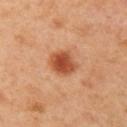follow-up: total-body-photography surveillance lesion; no biopsy
site: the left upper arm
automated metrics: a footprint of about 8 mm², a shape eccentricity near 0.5, and two-axis asymmetry of about 0.2; about 14 CIELAB-L* units darker than the surrounding skin; a border-irregularity rating of about 2/10 and internal color variation of about 4.5 on a 0–10 scale; a nevus-likeness score of about 100/100 and lesion-presence confidence of about 100/100
image: ~15 mm tile from a whole-body skin photo
patient: female, roughly 40 years of age